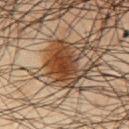Recorded during total-body skin imaging; not selected for excision or biopsy. A close-up tile cropped from a whole-body skin photograph, about 15 mm across. About 5.5 mm across. The tile uses cross-polarized illumination. A male patient, aged around 65. From the chest.